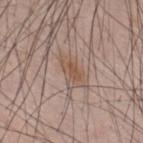{
  "biopsy_status": "not biopsied; imaged during a skin examination",
  "image": {
    "source": "total-body photography crop",
    "field_of_view_mm": 15
  },
  "site": "mid back",
  "patient": {
    "sex": "male",
    "age_approx": 35
  }
}